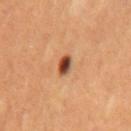follow-up: imaged on a skin check; not biopsied | patient: male, approximately 55 years of age | tile lighting: cross-polarized illumination | image source: ~15 mm tile from a whole-body skin photo | automated lesion analysis: a footprint of about 3.5 mm², an eccentricity of roughly 0.7, and a shape-asymmetry score of about 0.3 (0 = symmetric) | site: the mid back.